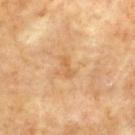Q: Was this lesion biopsied?
A: total-body-photography surveillance lesion; no biopsy
Q: Illumination type?
A: cross-polarized
Q: What is the lesion's diameter?
A: ≈2.5 mm
Q: What are the patient's age and sex?
A: about 60 years old
Q: Lesion location?
A: the upper back
Q: How was this image acquired?
A: 15 mm crop, total-body photography